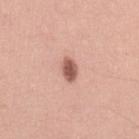Clinical impression:
Part of a total-body skin-imaging series; this lesion was reviewed on a skin check and was not flagged for biopsy.